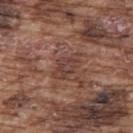notes: catalogued during a skin exam; not biopsied
subject: male, in their mid-70s
lesion diameter: ~4 mm (longest diameter)
acquisition: 15 mm crop, total-body photography
anatomic site: the back